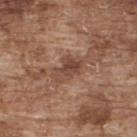biopsy status = imaged on a skin check; not biopsied | image = total-body-photography crop, ~15 mm field of view | lesion size = about 4 mm | patient = male, in their mid- to late 70s | site = the upper back | tile lighting = white-light.